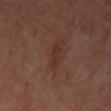Automated image analysis of the tile measured a shape eccentricity near 0.9 and a symmetry-axis asymmetry near 0.45. And it measured a mean CIELAB color near L≈32 a*≈21 b*≈25 and a normalized lesion–skin contrast near 6. The lesion is on the upper back. A 15 mm close-up tile from a total-body photography series done for melanoma screening. About 2.5 mm across. The patient is a female roughly 50 years of age. Captured under cross-polarized illumination.